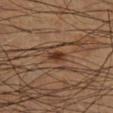Impression: The lesion was tiled from a total-body skin photograph and was not biopsied. Acquisition and patient details: The subject is a male aged 63 to 67. Longest diameter approximately 2.5 mm. The tile uses cross-polarized illumination. The lesion is located on the right lower leg. A 15 mm close-up tile from a total-body photography series done for melanoma screening.